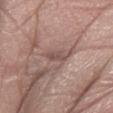anatomic site = the right forearm
image source = 15 mm crop, total-body photography
lesion size = ≈2.5 mm
illumination = white-light
patient = male, aged 78–82